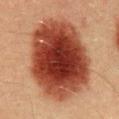{"biopsy_status": "not biopsied; imaged during a skin examination", "image": {"source": "total-body photography crop", "field_of_view_mm": 15}, "automated_metrics": {"cielab_L": 34, "cielab_a": 25, "cielab_b": 28, "vs_skin_darker_L": 18.0, "vs_skin_contrast_norm": 14.5}, "lighting": "cross-polarized", "site": "abdomen", "patient": {"sex": "male", "age_approx": 40}}